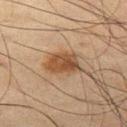<case>
<biopsy_status>not biopsied; imaged during a skin examination</biopsy_status>
<patient>
  <sex>male</sex>
  <age_approx>65</age_approx>
</patient>
<image>
  <source>total-body photography crop</source>
  <field_of_view_mm>15</field_of_view_mm>
</image>
<site>leg</site>
</case>